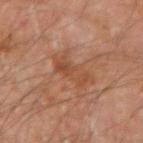biopsy_status: not biopsied; imaged during a skin examination
image:
  source: total-body photography crop
  field_of_view_mm: 15
lesion_size:
  long_diameter_mm_approx: 4.5
lighting: cross-polarized
site: left forearm
automated_metrics:
  area_mm2_approx: 7.5
  eccentricity: 0.9
  shape_asymmetry: 0.3
  color_variation_0_10: 4.0
  peripheral_color_asymmetry: 1.5
  nevus_likeness_0_100: 0
  lesion_detection_confidence_0_100: 100
patient:
  sex: male
  age_approx: 45